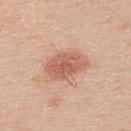Clinical impression: No biopsy was performed on this lesion — it was imaged during a full skin examination and was not determined to be concerning. Background: The lesion's longest dimension is about 5 mm. The lesion is on the upper back. A male patient, aged approximately 45. A close-up tile cropped from a whole-body skin photograph, about 15 mm across. This is a white-light tile.